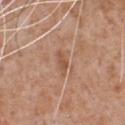{
  "biopsy_status": "not biopsied; imaged during a skin examination",
  "site": "chest",
  "image": {
    "source": "total-body photography crop",
    "field_of_view_mm": 15
  },
  "lesion_size": {
    "long_diameter_mm_approx": 3.0
  },
  "automated_metrics": {
    "area_mm2_approx": 3.0,
    "eccentricity": 0.9,
    "shape_asymmetry": 0.25,
    "border_irregularity_0_10": 2.5,
    "color_variation_0_10": 1.0,
    "peripheral_color_asymmetry": 0.5
  },
  "patient": {
    "sex": "male",
    "age_approx": 65
  }
}